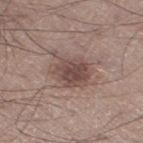Notes:
- biopsy status: total-body-photography surveillance lesion; no biopsy
- subject: male, aged 53 to 57
- acquisition: 15 mm crop, total-body photography
- tile lighting: white-light
- location: the right thigh